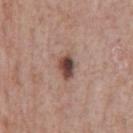This image is a 15 mm lesion crop taken from a total-body photograph. The patient is a male in their 70s. The lesion is located on the chest.